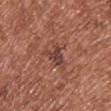Impression:
Recorded during total-body skin imaging; not selected for excision or biopsy.
Background:
Longest diameter approximately 3 mm. Captured under white-light illumination. From the chest. This image is a 15 mm lesion crop taken from a total-body photograph. The subject is a female approximately 40 years of age.